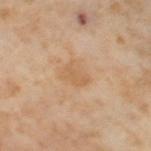The lesion was tiled from a total-body skin photograph and was not biopsied. The subject is a female in their mid- to late 50s. Measured at roughly 3 mm in maximum diameter. A 15 mm close-up tile from a total-body photography series done for melanoma screening. This is a cross-polarized tile. The lesion is on the right thigh.